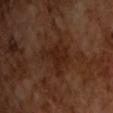Imaged during a routine full-body skin examination; the lesion was not biopsied and no histopathology is available.
A male subject, in their mid- to late 60s.
This image is a 15 mm lesion crop taken from a total-body photograph.